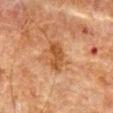workup = catalogued during a skin exam; not biopsied
anatomic site = the abdomen
imaging modality = ~15 mm crop, total-body skin-cancer survey
tile lighting = cross-polarized
patient = male, approximately 80 years of age